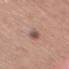Captured during whole-body skin photography for melanoma surveillance; the lesion was not biopsied. A female patient roughly 50 years of age. A lesion tile, about 15 mm wide, cut from a 3D total-body photograph. The recorded lesion diameter is about 3.5 mm. From the right forearm.